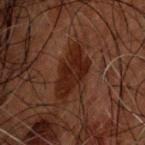This lesion was catalogued during total-body skin photography and was not selected for biopsy.
The subject is a male aged 48–52.
The lesion is located on the head or neck.
Automated tile analysis of the lesion measured a nevus-likeness score of about 85/100 and a lesion-detection confidence of about 95/100.
A lesion tile, about 15 mm wide, cut from a 3D total-body photograph.